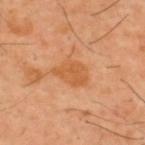The lesion is on the upper back. The subject is a male aged 38–42. This is a cross-polarized tile. Measured at roughly 4 mm in maximum diameter. A region of skin cropped from a whole-body photographic capture, roughly 15 mm wide.